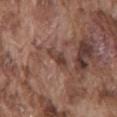Q: Was this lesion biopsied?
A: catalogued during a skin exam; not biopsied
Q: What are the patient's age and sex?
A: male, aged approximately 75
Q: What kind of image is this?
A: ~15 mm crop, total-body skin-cancer survey
Q: Illumination type?
A: white-light
Q: Where on the body is the lesion?
A: the back
Q: What is the lesion's diameter?
A: about 3 mm